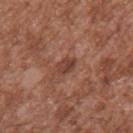acquisition: 15 mm crop, total-body photography; body site: the arm; subject: male, aged 43 to 47.